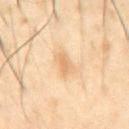The lesion was tiled from a total-body skin photograph and was not biopsied. The patient is a male aged around 50. On the abdomen. Longest diameter approximately 3 mm. A lesion tile, about 15 mm wide, cut from a 3D total-body photograph.